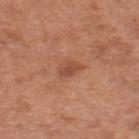Captured during whole-body skin photography for melanoma surveillance; the lesion was not biopsied. Imaged with white-light lighting. Measured at roughly 2.5 mm in maximum diameter. On the upper back. A close-up tile cropped from a whole-body skin photograph, about 15 mm across. A male patient, in their mid-60s.